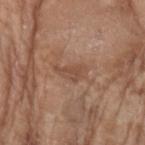follow-up=no biopsy performed (imaged during a skin exam) | lesion size=≈3.5 mm | image source=15 mm crop, total-body photography | body site=the right forearm | patient=female, roughly 75 years of age | image-analysis metrics=an eccentricity of roughly 0.85; about 7 CIELAB-L* units darker than the surrounding skin and a normalized lesion–skin contrast near 5.5.